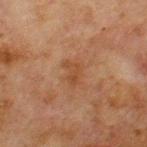workup — imaged on a skin check; not biopsied
patient — male, aged 63 to 67
diameter — ~3 mm (longest diameter)
anatomic site — the chest
image — ~15 mm tile from a whole-body skin photo
TBP lesion metrics — a footprint of about 4 mm², an eccentricity of roughly 0.8, and two-axis asymmetry of about 0.35; internal color variation of about 2 on a 0–10 scale and a peripheral color-asymmetry measure near 0.5; lesion-presence confidence of about 100/100